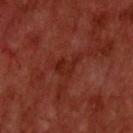biopsy status=no biopsy performed (imaged during a skin exam); patient=male, about 60 years old; image source=~15 mm tile from a whole-body skin photo; lesion size=about 3.5 mm; illumination=cross-polarized illumination; location=the upper back.